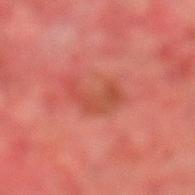Assessment:
Part of a total-body skin-imaging series; this lesion was reviewed on a skin check and was not flagged for biopsy.
Context:
A 15 mm crop from a total-body photograph taken for skin-cancer surveillance. A male subject, about 75 years old. This is a cross-polarized tile. Located on the left lower leg. Automated image analysis of the tile measured a border-irregularity rating of about 7.5/10, a color-variation rating of about 2.5/10, and a peripheral color-asymmetry measure near 1. The analysis additionally found an automated nevus-likeness rating near 0 out of 100. About 5 mm across.